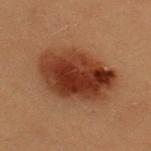Imaged during a routine full-body skin examination; the lesion was not biopsied and no histopathology is available. Approximately 8.5 mm at its widest. A female patient, aged around 20. The tile uses cross-polarized illumination. A close-up tile cropped from a whole-body skin photograph, about 15 mm across. The lesion-visualizer software estimated a footprint of about 34 mm², an eccentricity of roughly 0.8, and a shape-asymmetry score of about 0.15 (0 = symmetric). It also reported an average lesion color of about L≈28 a*≈21 b*≈26 (CIELAB), roughly 12 lightness units darker than nearby skin, and a normalized border contrast of about 12. It also reported a color-variation rating of about 7/10 and a peripheral color-asymmetry measure near 3. And it measured a classifier nevus-likeness of about 100/100 and a lesion-detection confidence of about 100/100. From the back.